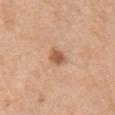Case summary:
* biopsy status · total-body-photography surveillance lesion; no biopsy
* acquisition · total-body-photography crop, ~15 mm field of view
* location · the chest
* size · about 2.5 mm
* patient · female, about 55 years old
* TBP lesion metrics · an outline eccentricity of about 0.6 (0 = round, 1 = elongated) and a symmetry-axis asymmetry near 0.2; an average lesion color of about L≈57 a*≈22 b*≈35 (CIELAB), roughly 12 lightness units darker than nearby skin, and a normalized lesion–skin contrast near 8; border irregularity of about 1.5 on a 0–10 scale; an automated nevus-likeness rating near 90 out of 100